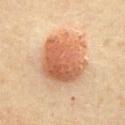No biopsy was performed on this lesion — it was imaged during a full skin examination and was not determined to be concerning. Measured at roughly 6.5 mm in maximum diameter. Captured under cross-polarized illumination. A male patient aged 68 to 72. A region of skin cropped from a whole-body photographic capture, roughly 15 mm wide. The lesion is on the chest. Automated tile analysis of the lesion measured border irregularity of about 2.5 on a 0–10 scale, internal color variation of about 6.5 on a 0–10 scale, and a peripheral color-asymmetry measure near 2.